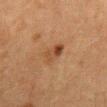Q: Lesion location?
A: the abdomen
Q: What kind of image is this?
A: total-body-photography crop, ~15 mm field of view
Q: What lighting was used for the tile?
A: cross-polarized illumination
Q: Lesion size?
A: ≈3 mm
Q: What did automated image analysis measure?
A: a mean CIELAB color near L≈38 a*≈19 b*≈30, a lesion–skin lightness drop of about 8, and a lesion-to-skin contrast of about 7 (normalized; higher = more distinct)
Q: Patient demographics?
A: female, in their 50s